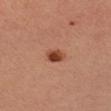Q: Was this lesion biopsied?
A: catalogued during a skin exam; not biopsied
Q: Who is the patient?
A: female, in their mid-30s
Q: What is the lesion's diameter?
A: ~2.5 mm (longest diameter)
Q: Where on the body is the lesion?
A: the left forearm
Q: What lighting was used for the tile?
A: cross-polarized illumination
Q: How was this image acquired?
A: total-body-photography crop, ~15 mm field of view